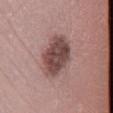No biopsy was performed on this lesion — it was imaged during a full skin examination and was not determined to be concerning.
The subject is a female roughly 40 years of age.
The lesion is located on the right lower leg.
This image is a 15 mm lesion crop taken from a total-body photograph.
Imaged with white-light lighting.
The lesion's longest dimension is about 5.5 mm.